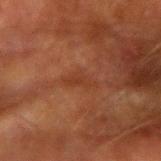| feature | finding |
|---|---|
| subject | male, in their mid- to late 60s |
| illumination | cross-polarized |
| location | the left forearm |
| image source | ~15 mm tile from a whole-body skin photo |
| lesion size | ≈3.5 mm |